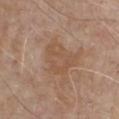Clinical impression:
Captured during whole-body skin photography for melanoma surveillance; the lesion was not biopsied.
Context:
The subject is a male roughly 80 years of age. This image is a 15 mm lesion crop taken from a total-body photograph. The lesion-visualizer software estimated an automated nevus-likeness rating near 0 out of 100. Located on the front of the torso. Approximately 4.5 mm at its widest.